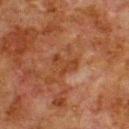Recorded during total-body skin imaging; not selected for excision or biopsy.
The total-body-photography lesion software estimated a mean CIELAB color near L≈33 a*≈21 b*≈30 and roughly 6 lightness units darker than nearby skin.
The subject is a male aged approximately 80.
Cropped from a total-body skin-imaging series; the visible field is about 15 mm.
On the upper back.
This is a cross-polarized tile.